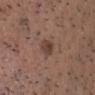Part of a total-body skin-imaging series; this lesion was reviewed on a skin check and was not flagged for biopsy. The patient is a male approximately 65 years of age. The recorded lesion diameter is about 2.5 mm. Located on the chest. Imaged with white-light lighting. This image is a 15 mm lesion crop taken from a total-body photograph.